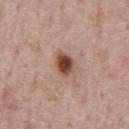Findings:
• workup · total-body-photography surveillance lesion; no biopsy